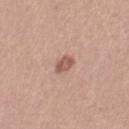workup=catalogued during a skin exam; not biopsied | diameter=~2.5 mm (longest diameter) | illumination=white-light illumination | patient=female, in their mid-50s | body site=the right thigh | image-analysis metrics=a lesion area of about 4 mm², an eccentricity of roughly 0.7, and a symmetry-axis asymmetry near 0.3; a lesion color around L≈56 a*≈22 b*≈26 in CIELAB, a lesion–skin lightness drop of about 11, and a normalized lesion–skin contrast near 7.5 | acquisition=total-body-photography crop, ~15 mm field of view.